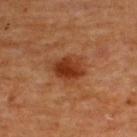Part of a total-body skin-imaging series; this lesion was reviewed on a skin check and was not flagged for biopsy. A close-up tile cropped from a whole-body skin photograph, about 15 mm across. A male subject aged approximately 65. Approximately 4 mm at its widest. Located on the upper back.